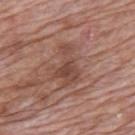Q: Was a biopsy performed?
A: catalogued during a skin exam; not biopsied
Q: Lesion location?
A: the mid back
Q: What are the patient's age and sex?
A: male, aged approximately 70
Q: What lighting was used for the tile?
A: white-light illumination
Q: How large is the lesion?
A: ~5 mm (longest diameter)
Q: What is the imaging modality?
A: ~15 mm tile from a whole-body skin photo
Q: Automated lesion metrics?
A: a lesion area of about 9.5 mm², an outline eccentricity of about 0.85 (0 = round, 1 = elongated), and a shape-asymmetry score of about 0.5 (0 = symmetric); a lesion color around L≈46 a*≈21 b*≈25 in CIELAB, about 9 CIELAB-L* units darker than the surrounding skin, and a normalized lesion–skin contrast near 6.5; border irregularity of about 6.5 on a 0–10 scale, internal color variation of about 3 on a 0–10 scale, and a peripheral color-asymmetry measure near 1